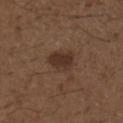Recorded during total-body skin imaging; not selected for excision or biopsy.
On the chest.
A 15 mm close-up extracted from a 3D total-body photography capture.
The tile uses white-light illumination.
Longest diameter approximately 3.5 mm.
The patient is a male aged around 50.